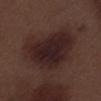<record>
<biopsy_status>not biopsied; imaged during a skin examination</biopsy_status>
<image>
  <source>total-body photography crop</source>
  <field_of_view_mm>15</field_of_view_mm>
</image>
<automated_metrics>
  <area_mm2_approx>35.0</area_mm2_approx>
  <eccentricity>0.7</eccentricity>
  <shape_asymmetry>0.3</shape_asymmetry>
  <cielab_L>23</cielab_L>
  <cielab_a>16</cielab_a>
  <cielab_b>16</cielab_b>
  <vs_skin_darker_L>8.0</vs_skin_darker_L>
  <vs_skin_contrast_norm>10.5</vs_skin_contrast_norm>
  <border_irregularity_0_10>3.0</border_irregularity_0_10>
  <peripheral_color_asymmetry>1.0</peripheral_color_asymmetry>
  <nevus_likeness_0_100>85</nevus_likeness_0_100>
  <lesion_detection_confidence_0_100>100</lesion_detection_confidence_0_100>
</automated_metrics>
<lesion_size>
  <long_diameter_mm_approx>8.0</long_diameter_mm_approx>
</lesion_size>
<patient>
  <sex>male</sex>
  <age_approx>70</age_approx>
</patient>
<site>left lower leg</site>
<lighting>white-light</lighting>
</record>